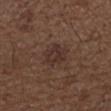Clinical impression:
No biopsy was performed on this lesion — it was imaged during a full skin examination and was not determined to be concerning.
Image and clinical context:
Captured under white-light illumination. The patient is a male aged around 50. A 15 mm close-up extracted from a 3D total-body photography capture. The lesion is located on the right forearm.